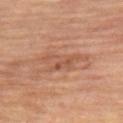subject: female, aged 68 to 72
image source: 15 mm crop, total-body photography
tile lighting: cross-polarized
location: the leg
automated lesion analysis: a color-variation rating of about 4/10 and radial color variation of about 1.5; a classifier nevus-likeness of about 0/100 and a detector confidence of about 95 out of 100 that the crop contains a lesion
lesion diameter: ~5.5 mm (longest diameter)